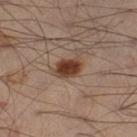The lesion was photographed on a routine skin check and not biopsied; there is no pathology result. Approximately 3 mm at its widest. The lesion is located on the left leg. This image is a 15 mm lesion crop taken from a total-body photograph. The subject is a male approximately 50 years of age.